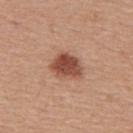biopsy status=catalogued during a skin exam; not biopsied
diameter=≈4 mm
TBP lesion metrics=a lesion–skin lightness drop of about 15 and a normalized border contrast of about 10; a within-lesion color-variation index near 3.5/10; an automated nevus-likeness rating near 100 out of 100 and lesion-presence confidence of about 100/100
subject=male, roughly 55 years of age
lighting=white-light
image source=total-body-photography crop, ~15 mm field of view
location=the upper back